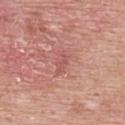Clinical impression:
The lesion was photographed on a routine skin check and not biopsied; there is no pathology result.
Clinical summary:
Located on the upper back. The total-body-photography lesion software estimated an outline eccentricity of about 0.85 (0 = round, 1 = elongated) and two-axis asymmetry of about 0.25. It also reported a border-irregularity index near 3.5/10 and a peripheral color-asymmetry measure near 1. And it measured a nevus-likeness score of about 0/100 and lesion-presence confidence of about 100/100. Cropped from a total-body skin-imaging series; the visible field is about 15 mm. The lesion's longest dimension is about 3 mm. A male subject roughly 60 years of age.